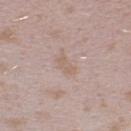Imaged during a routine full-body skin examination; the lesion was not biopsied and no histopathology is available.
A female subject aged 23 to 27.
The lesion's longest dimension is about 3 mm.
Cropped from a total-body skin-imaging series; the visible field is about 15 mm.
This is a white-light tile.
The lesion is on the right lower leg.